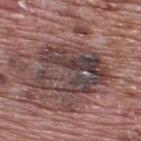Q: Was this lesion biopsied?
A: no biopsy performed (imaged during a skin exam)
Q: Where on the body is the lesion?
A: the upper back
Q: How was the tile lit?
A: white-light
Q: Who is the patient?
A: male, about 70 years old
Q: What is the imaging modality?
A: 15 mm crop, total-body photography
Q: How large is the lesion?
A: ≈8 mm